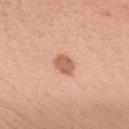Case summary:
- notes — catalogued during a skin exam; not biopsied
- anatomic site — the upper back
- lesion size — ~3 mm (longest diameter)
- lighting — white-light
- patient — male, in their mid- to late 50s
- image-analysis metrics — an area of roughly 5 mm², an outline eccentricity of about 0.75 (0 = round, 1 = elongated), and two-axis asymmetry of about 0.15; a border-irregularity index near 1.5/10, a within-lesion color-variation index near 1.5/10, and a peripheral color-asymmetry measure near 0.5; an automated nevus-likeness rating near 90 out of 100
- image — ~15 mm tile from a whole-body skin photo